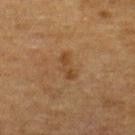Findings:
* biopsy status — catalogued during a skin exam; not biopsied